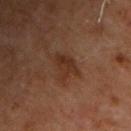Context: A male subject aged around 70. The total-body-photography lesion software estimated a footprint of about 6 mm², an outline eccentricity of about 0.75 (0 = round, 1 = elongated), and a symmetry-axis asymmetry near 0.4. The software also gave a lesion-to-skin contrast of about 7 (normalized; higher = more distinct). And it measured a within-lesion color-variation index near 2/10 and peripheral color asymmetry of about 1. The analysis additionally found a classifier nevus-likeness of about 15/100 and a lesion-detection confidence of about 100/100. The lesion is on the chest. The recorded lesion diameter is about 3.5 mm. A 15 mm close-up extracted from a 3D total-body photography capture.